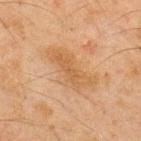Clinical impression: Imaged during a routine full-body skin examination; the lesion was not biopsied and no histopathology is available. Acquisition and patient details: A male patient, aged approximately 45. The recorded lesion diameter is about 6.5 mm. Cropped from a total-body skin-imaging series; the visible field is about 15 mm. Located on the back. Imaged with cross-polarized lighting. The total-body-photography lesion software estimated a shape eccentricity near 0.9 and a shape-asymmetry score of about 0.4 (0 = symmetric). It also reported a border-irregularity index near 5/10.